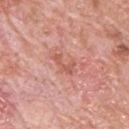{
  "biopsy_status": "not biopsied; imaged during a skin examination",
  "site": "upper back",
  "patient": {
    "sex": "male",
    "age_approx": 80
  },
  "image": {
    "source": "total-body photography crop",
    "field_of_view_mm": 15
  }
}